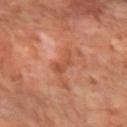site — the left forearm | subject — female, aged approximately 65 | image — ~15 mm tile from a whole-body skin photo.